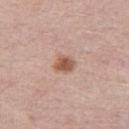The lesion was photographed on a routine skin check and not biopsied; there is no pathology result. The lesion is located on the right thigh. A female patient approximately 45 years of age. A 15 mm crop from a total-body photograph taken for skin-cancer surveillance.